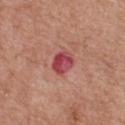Findings:
• workup · total-body-photography surveillance lesion; no biopsy
• tile lighting · white-light illumination
• location · the upper back
• lesion diameter · ~3 mm (longest diameter)
• image source · total-body-photography crop, ~15 mm field of view
• patient · male, aged 78–82